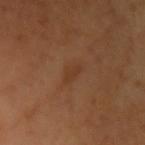Notes:
– biopsy status — imaged on a skin check; not biopsied
– site — the left upper arm
– subject — female, in their mid-50s
– acquisition — 15 mm crop, total-body photography